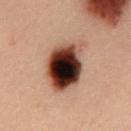patient:
  sex: male
  age_approx: 40
lesion_size:
  long_diameter_mm_approx: 7.0
image:
  source: total-body photography crop
  field_of_view_mm: 15
site: abdomen
lighting: cross-polarized
diagnosis:
  histopathology: invasive melanoma
  malignancy: malignant
  taxonomic_path:
    - Malignant
    - Malignant melanocytic proliferations (Melanoma)
    - Melanoma Invasive
  breslow_mm: 0.55
  mitotic_index: 0/mm²